A 15 mm close-up tile from a total-body photography series done for melanoma screening, a male subject aged 68–72, located on the chest: 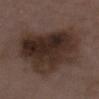{
  "lighting": "white-light",
  "lesion_size": {
    "long_diameter_mm_approx": 9.5
  },
  "automated_metrics": {
    "vs_skin_darker_L": 11.0,
    "vs_skin_contrast_norm": 11.0,
    "border_irregularity_0_10": 3.0,
    "peripheral_color_asymmetry": 2.5
  },
  "diagnosis": {
    "histopathology": "seborrheic keratosis",
    "malignancy": "benign",
    "taxonomic_path": [
      "Benign",
      "Benign epidermal proliferations",
      "Seborrheic keratosis"
    ]
  }
}